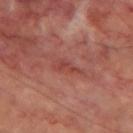notes: catalogued during a skin exam; not biopsied
subject: male, roughly 70 years of age
illumination: cross-polarized
lesion diameter: about 3.5 mm
image-analysis metrics: a lesion area of about 4 mm², an outline eccentricity of about 0.9 (0 = round, 1 = elongated), and a shape-asymmetry score of about 0.45 (0 = symmetric); a mean CIELAB color near L≈43 a*≈28 b*≈25, about 7 CIELAB-L* units darker than the surrounding skin, and a lesion-to-skin contrast of about 5.5 (normalized; higher = more distinct); a classifier nevus-likeness of about 0/100
location: the left thigh
image: total-body-photography crop, ~15 mm field of view